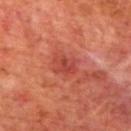Clinical impression: No biopsy was performed on this lesion — it was imaged during a full skin examination and was not determined to be concerning. Context: Automated tile analysis of the lesion measured a lesion area of about 5.5 mm² and a symmetry-axis asymmetry near 0.25. And it measured border irregularity of about 3 on a 0–10 scale. The patient is a male about 70 years old. Measured at roughly 3 mm in maximum diameter. A 15 mm close-up extracted from a 3D total-body photography capture. The lesion is located on the mid back. Imaged with cross-polarized lighting.